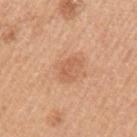| field | value |
|---|---|
| workup | no biopsy performed (imaged during a skin exam) |
| imaging modality | 15 mm crop, total-body photography |
| patient | male, aged 48 to 52 |
| illumination | white-light illumination |
| site | the left upper arm |
| diameter | about 3 mm |